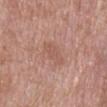* workup: catalogued during a skin exam; not biopsied
* image-analysis metrics: an automated nevus-likeness rating near 0 out of 100 and lesion-presence confidence of about 100/100
* patient: male, aged approximately 65
* location: the mid back
* image source: ~15 mm crop, total-body skin-cancer survey
* lesion size: about 5 mm
* tile lighting: white-light